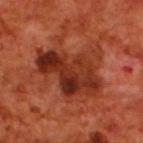Findings:
• follow-up · total-body-photography surveillance lesion; no biopsy
• subject · male, approximately 70 years of age
• location · the upper back
• lesion diameter · ~7 mm (longest diameter)
• image source · total-body-photography crop, ~15 mm field of view
• lighting · cross-polarized
• automated metrics · border irregularity of about 6.5 on a 0–10 scale, internal color variation of about 8.5 on a 0–10 scale, and a peripheral color-asymmetry measure near 3.5